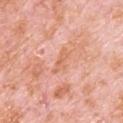notes = total-body-photography surveillance lesion; no biopsy | lesion size = about 3.5 mm | patient = male, aged around 80 | body site = the upper back | image = ~15 mm crop, total-body skin-cancer survey | automated lesion analysis = an eccentricity of roughly 0.9 and a symmetry-axis asymmetry near 0.3; a border-irregularity rating of about 4/10, internal color variation of about 0.5 on a 0–10 scale, and peripheral color asymmetry of about 0 | illumination = white-light illumination.